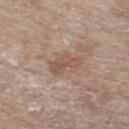workup: catalogued during a skin exam; not biopsied
automated metrics: a footprint of about 6.5 mm², an outline eccentricity of about 0.9 (0 = round, 1 = elongated), and a shape-asymmetry score of about 0.35 (0 = symmetric); a lesion color around L≈53 a*≈17 b*≈27 in CIELAB and a lesion–skin lightness drop of about 8
image source: ~15 mm crop, total-body skin-cancer survey
patient: female, roughly 75 years of age
lighting: white-light illumination
body site: the right thigh
lesion size: about 4 mm